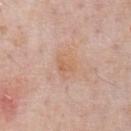No biopsy was performed on this lesion — it was imaged during a full skin examination and was not determined to be concerning. A male patient, in their 60s. Located on the front of the torso. A region of skin cropped from a whole-body photographic capture, roughly 15 mm wide. Measured at roughly 2.5 mm in maximum diameter. An algorithmic analysis of the crop reported an area of roughly 5 mm², an eccentricity of roughly 0.55, and a symmetry-axis asymmetry near 0.4. The software also gave a mean CIELAB color near L≈63 a*≈20 b*≈33, a lesion–skin lightness drop of about 6, and a normalized border contrast of about 5.5. And it measured a nevus-likeness score of about 0/100 and lesion-presence confidence of about 100/100.